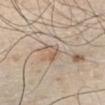Findings:
• follow-up — total-body-photography surveillance lesion; no biopsy
• subject — male, approximately 30 years of age
• body site — the chest
• tile lighting — white-light illumination
• acquisition — ~15 mm crop, total-body skin-cancer survey
• automated lesion analysis — a lesion area of about 4 mm², an eccentricity of roughly 0.8, and a shape-asymmetry score of about 0.4 (0 = symmetric); an average lesion color of about L≈59 a*≈14 b*≈28 (CIELAB), about 7 CIELAB-L* units darker than the surrounding skin, and a normalized lesion–skin contrast near 5.5; a nevus-likeness score of about 15/100 and a detector confidence of about 55 out of 100 that the crop contains a lesion
• size — ≈3 mm